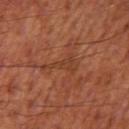Impression: No biopsy was performed on this lesion — it was imaged during a full skin examination and was not determined to be concerning. Clinical summary: The total-body-photography lesion software estimated a lesion color around L≈40 a*≈25 b*≈32 in CIELAB, about 6 CIELAB-L* units darker than the surrounding skin, and a normalized lesion–skin contrast near 5. It also reported radial color variation of about 0.5. It also reported a detector confidence of about 65 out of 100 that the crop contains a lesion. A male subject in their mid-50s. A roughly 15 mm field-of-view crop from a total-body skin photograph. The recorded lesion diameter is about 4.5 mm. From the right thigh.